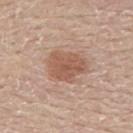{
  "biopsy_status": "not biopsied; imaged during a skin examination",
  "patient": {
    "sex": "male",
    "age_approx": 60
  },
  "lesion_size": {
    "long_diameter_mm_approx": 5.0
  },
  "automated_metrics": {
    "area_mm2_approx": 13.0,
    "shape_asymmetry": 0.25,
    "vs_skin_darker_L": 10.0,
    "vs_skin_contrast_norm": 7.0
  },
  "site": "upper back",
  "image": {
    "source": "total-body photography crop",
    "field_of_view_mm": 15
  },
  "lighting": "white-light"
}